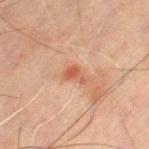{
  "biopsy_status": "not biopsied; imaged during a skin examination",
  "lighting": "cross-polarized",
  "automated_metrics": {
    "area_mm2_approx": 3.5,
    "eccentricity": 0.75,
    "shape_asymmetry": 0.5
  },
  "lesion_size": {
    "long_diameter_mm_approx": 2.5
  },
  "patient": {
    "sex": "male",
    "age_approx": 60
  },
  "site": "left thigh",
  "image": {
    "source": "total-body photography crop",
    "field_of_view_mm": 15
  }
}